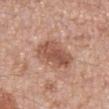{"biopsy_status": "not biopsied; imaged during a skin examination", "patient": {"sex": "female", "age_approx": 50}, "site": "arm", "image": {"source": "total-body photography crop", "field_of_view_mm": 15}}